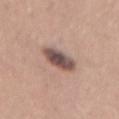Q: Was a biopsy performed?
A: total-body-photography surveillance lesion; no biopsy
Q: Illumination type?
A: white-light illumination
Q: Lesion size?
A: ≈3.5 mm
Q: What is the imaging modality?
A: 15 mm crop, total-body photography
Q: Who is the patient?
A: female, in their 30s
Q: What did automated image analysis measure?
A: an average lesion color of about L≈50 a*≈17 b*≈20 (CIELAB), roughly 16 lightness units darker than nearby skin, and a normalized border contrast of about 11.5; an automated nevus-likeness rating near 50 out of 100 and a detector confidence of about 100 out of 100 that the crop contains a lesion
Q: What is the anatomic site?
A: the lower back